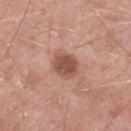Impression:
No biopsy was performed on this lesion — it was imaged during a full skin examination and was not determined to be concerning.
Background:
On the left lower leg. About 3.5 mm across. An algorithmic analysis of the crop reported a mean CIELAB color near L≈51 a*≈23 b*≈27, roughly 12 lightness units darker than nearby skin, and a normalized lesion–skin contrast near 8.5. It also reported a border-irregularity index near 1/10, a color-variation rating of about 3/10, and peripheral color asymmetry of about 1. It also reported a detector confidence of about 100 out of 100 that the crop contains a lesion. A male patient, roughly 50 years of age. A lesion tile, about 15 mm wide, cut from a 3D total-body photograph. Imaged with white-light lighting.